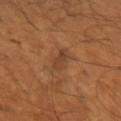Findings:
– site: the left upper arm
– tile lighting: cross-polarized illumination
– imaging modality: total-body-photography crop, ~15 mm field of view
– subject: male, roughly 55 years of age